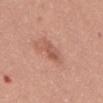Imaged during a routine full-body skin examination; the lesion was not biopsied and no histopathology is available.
A close-up tile cropped from a whole-body skin photograph, about 15 mm across.
The lesion is located on the abdomen.
The recorded lesion diameter is about 3 mm.
A female patient, about 35 years old.